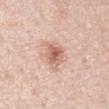No biopsy was performed on this lesion — it was imaged during a full skin examination and was not determined to be concerning.
The subject is a male in their 60s.
Cropped from a whole-body photographic skin survey; the tile spans about 15 mm.
The lesion is located on the arm.